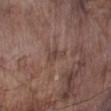workup: catalogued during a skin exam; not biopsied | patient: male, aged approximately 75 | lighting: white-light illumination | acquisition: 15 mm crop, total-body photography | diameter: about 2.5 mm | anatomic site: the left lower leg.